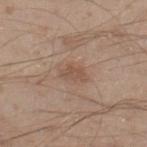Clinical impression: No biopsy was performed on this lesion — it was imaged during a full skin examination and was not determined to be concerning. Acquisition and patient details: The total-body-photography lesion software estimated an eccentricity of roughly 0.85 and a shape-asymmetry score of about 0.35 (0 = symmetric). It also reported a lesion–skin lightness drop of about 7 and a normalized border contrast of about 5.5. And it measured a color-variation rating of about 1/10 and peripheral color asymmetry of about 0.5. The analysis additionally found a lesion-detection confidence of about 100/100. The lesion is on the right lower leg. The patient is a male aged 33 to 37. The tile uses white-light illumination. Measured at roughly 3 mm in maximum diameter. Cropped from a whole-body photographic skin survey; the tile spans about 15 mm.